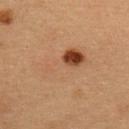Clinical impression: This lesion was catalogued during total-body skin photography and was not selected for biopsy. Image and clinical context: This is a cross-polarized tile. The lesion-visualizer software estimated a lesion area of about 20 mm², an eccentricity of roughly 0.85, and two-axis asymmetry of about 0.25. A female subject about 30 years old. On the upper back. This image is a 15 mm lesion crop taken from a total-body photograph. About 7 mm across.